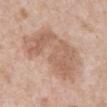<tbp_lesion>
<biopsy_status>not biopsied; imaged during a skin examination</biopsy_status>
<image>
  <source>total-body photography crop</source>
  <field_of_view_mm>15</field_of_view_mm>
</image>
<lesion_size>
  <long_diameter_mm_approx>8.0</long_diameter_mm_approx>
</lesion_size>
<patient>
  <sex>male</sex>
  <age_approx>80</age_approx>
</patient>
<site>front of the torso</site>
<lighting>white-light</lighting>
</tbp_lesion>